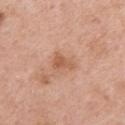Q: What is the anatomic site?
A: the right upper arm
Q: Patient demographics?
A: female, aged approximately 40
Q: What kind of image is this?
A: ~15 mm crop, total-body skin-cancer survey